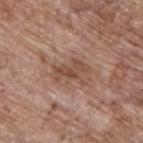notes: total-body-photography surveillance lesion; no biopsy | subject: male, approximately 70 years of age | automated metrics: an area of roughly 7 mm² and a shape-asymmetry score of about 0.35 (0 = symmetric); a lesion color around L≈48 a*≈20 b*≈27 in CIELAB, about 9 CIELAB-L* units darker than the surrounding skin, and a normalized border contrast of about 7; border irregularity of about 6 on a 0–10 scale and peripheral color asymmetry of about 1 | location: the upper back | illumination: white-light | image: 15 mm crop, total-body photography.